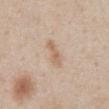Findings:
- workup — imaged on a skin check; not biopsied
- acquisition — ~15 mm tile from a whole-body skin photo
- anatomic site — the abdomen
- patient — male, aged 58–62